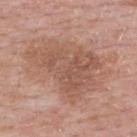No biopsy was performed on this lesion — it was imaged during a full skin examination and was not determined to be concerning.
An algorithmic analysis of the crop reported a color-variation rating of about 3.5/10 and radial color variation of about 1. It also reported a classifier nevus-likeness of about 0/100.
A male patient about 55 years old.
A lesion tile, about 15 mm wide, cut from a 3D total-body photograph.
Located on the upper back.
Captured under white-light illumination.